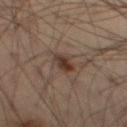Imaged during a routine full-body skin examination; the lesion was not biopsied and no histopathology is available. The patient is a male aged 53–57. A 15 mm crop from a total-body photograph taken for skin-cancer surveillance. The lesion is on the left thigh.